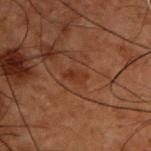biopsy status — no biopsy performed (imaged during a skin exam) | lighting — cross-polarized | imaging modality — 15 mm crop, total-body photography | TBP lesion metrics — a footprint of about 3 mm² and two-axis asymmetry of about 0.2; an average lesion color of about L≈25 a*≈19 b*≈25 (CIELAB), a lesion–skin lightness drop of about 5, and a lesion-to-skin contrast of about 5.5 (normalized; higher = more distinct); a detector confidence of about 100 out of 100 that the crop contains a lesion | body site — the back | size — ≈3 mm | patient — male, aged approximately 50.